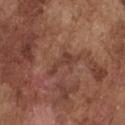workup: no biopsy performed (imaged during a skin exam) | size: ~4 mm (longest diameter) | body site: the front of the torso | image-analysis metrics: an area of roughly 4 mm² and two-axis asymmetry of about 0.55; a mean CIELAB color near L≈40 a*≈21 b*≈26, a lesion–skin lightness drop of about 7, and a lesion-to-skin contrast of about 6 (normalized; higher = more distinct); a border-irregularity rating of about 7/10 and peripheral color asymmetry of about 0.5 | imaging modality: total-body-photography crop, ~15 mm field of view | lighting: white-light | patient: male, aged 73–77.